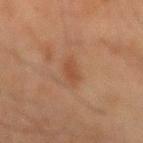Clinical impression:
This lesion was catalogued during total-body skin photography and was not selected for biopsy.